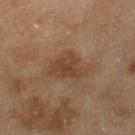Q: What is the lesion's diameter?
A: ~5 mm (longest diameter)
Q: How was this image acquired?
A: 15 mm crop, total-body photography
Q: What is the anatomic site?
A: the leg
Q: What are the patient's age and sex?
A: female, roughly 60 years of age